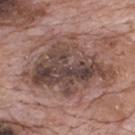workup = catalogued during a skin exam; not biopsied
illumination = white-light
image source = ~15 mm tile from a whole-body skin photo
lesion diameter = ≈10.5 mm
patient = male, aged 68 to 72
site = the back
image-analysis metrics = a lesion color around L≈45 a*≈17 b*≈21 in CIELAB, roughly 13 lightness units darker than nearby skin, and a normalized border contrast of about 9.5; border irregularity of about 6.5 on a 0–10 scale and a color-variation rating of about 8.5/10; an automated nevus-likeness rating near 5 out of 100